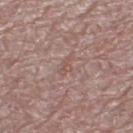notes = catalogued during a skin exam; not biopsied | anatomic site = the left thigh | subject = female, approximately 65 years of age | acquisition = total-body-photography crop, ~15 mm field of view.